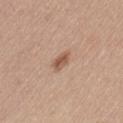biopsy status: total-body-photography surveillance lesion; no biopsy | tile lighting: white-light illumination | location: the lower back | imaging modality: 15 mm crop, total-body photography | patient: female, aged approximately 55 | size: ≈2.5 mm.